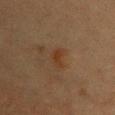Part of a total-body skin-imaging series; this lesion was reviewed on a skin check and was not flagged for biopsy. A female patient, aged approximately 45. The lesion is located on the chest. The tile uses cross-polarized illumination. A lesion tile, about 15 mm wide, cut from a 3D total-body photograph. The lesion's longest dimension is about 2.5 mm.